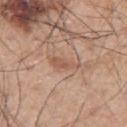Impression:
Part of a total-body skin-imaging series; this lesion was reviewed on a skin check and was not flagged for biopsy.
Clinical summary:
The lesion is located on the left arm. A male subject, in their 70s. About 3.5 mm across. Automated tile analysis of the lesion measured a lesion area of about 4 mm². The software also gave a mean CIELAB color near L≈55 a*≈20 b*≈29, about 8 CIELAB-L* units darker than the surrounding skin, and a lesion-to-skin contrast of about 5.5 (normalized; higher = more distinct). It also reported a nevus-likeness score of about 0/100. This is a white-light tile. Cropped from a whole-body photographic skin survey; the tile spans about 15 mm.